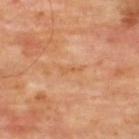| field | value |
|---|---|
| biopsy status | total-body-photography surveillance lesion; no biopsy |
| site | the upper back |
| acquisition | ~15 mm crop, total-body skin-cancer survey |
| patient | male, approximately 65 years of age |
| illumination | cross-polarized |
| automated lesion analysis | a shape eccentricity near 0.95; border irregularity of about 5 on a 0–10 scale, internal color variation of about 0 on a 0–10 scale, and radial color variation of about 0 |